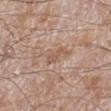• automated lesion analysis · a border-irregularity rating of about 4/10, internal color variation of about 0 on a 0–10 scale, and radial color variation of about 0; a classifier nevus-likeness of about 0/100 and a detector confidence of about 100 out of 100 that the crop contains a lesion
• patient · male, roughly 60 years of age
• illumination · white-light illumination
• lesion diameter · ≈3 mm
• imaging modality · total-body-photography crop, ~15 mm field of view
• anatomic site · the leg